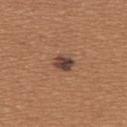Recorded during total-body skin imaging; not selected for excision or biopsy. The lesion is located on the back. A female subject, aged approximately 40. Captured under white-light illumination. This image is a 15 mm lesion crop taken from a total-body photograph.